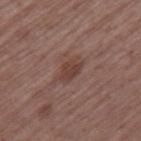{
  "patient": {
    "sex": "male",
    "age_approx": 65
  },
  "lighting": "white-light",
  "site": "right thigh",
  "image": {
    "source": "total-body photography crop",
    "field_of_view_mm": 15
  }
}